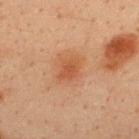workup = no biopsy performed (imaged during a skin exam)
patient = male, aged 33 to 37
size = ≈3 mm
image-analysis metrics = a border-irregularity rating of about 2/10, internal color variation of about 2 on a 0–10 scale, and radial color variation of about 0.5; a nevus-likeness score of about 40/100 and a detector confidence of about 100 out of 100 that the crop contains a lesion
location = the upper back
acquisition = total-body-photography crop, ~15 mm field of view
tile lighting = cross-polarized illumination